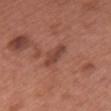* biopsy status · imaged on a skin check; not biopsied
* TBP lesion metrics · a footprint of about 4.5 mm²; a mean CIELAB color near L≈43 a*≈25 b*≈27, a lesion–skin lightness drop of about 9, and a lesion-to-skin contrast of about 7 (normalized; higher = more distinct)
* site · the chest
* subject · female, aged 58–62
* acquisition · ~15 mm crop, total-body skin-cancer survey
* tile lighting · white-light illumination